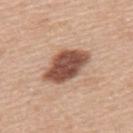Captured during whole-body skin photography for melanoma surveillance; the lesion was not biopsied. The lesion's longest dimension is about 6 mm. The tile uses white-light illumination. On the back. A male patient approximately 50 years of age. A close-up tile cropped from a whole-body skin photograph, about 15 mm across.